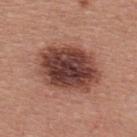Captured during whole-body skin photography for melanoma surveillance; the lesion was not biopsied. The patient is a male aged 38–42. The tile uses white-light illumination. Automated tile analysis of the lesion measured a border-irregularity index near 1.5/10, internal color variation of about 7.5 on a 0–10 scale, and a peripheral color-asymmetry measure near 2.5. The lesion is located on the upper back. A 15 mm crop from a total-body photograph taken for skin-cancer surveillance.